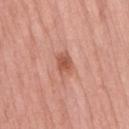Clinical impression: The lesion was tiled from a total-body skin photograph and was not biopsied. Context: The tile uses white-light illumination. The patient is a female aged 68–72. The total-body-photography lesion software estimated a lesion area of about 5 mm², an eccentricity of roughly 0.75, and a shape-asymmetry score of about 0.15 (0 = symmetric). And it measured a border-irregularity rating of about 1.5/10, internal color variation of about 3 on a 0–10 scale, and peripheral color asymmetry of about 1. A 15 mm crop from a total-body photograph taken for skin-cancer surveillance. From the lower back.